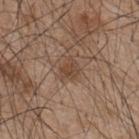biopsy_status: not biopsied; imaged during a skin examination
automated_metrics:
  area_mm2_approx: 5.0
  eccentricity: 0.45
  shape_asymmetry: 0.45
  vs_skin_contrast_norm: 6.5
lighting: white-light
lesion_size:
  long_diameter_mm_approx: 3.0
patient:
  sex: male
  age_approx: 45
site: back
image:
  source: total-body photography crop
  field_of_view_mm: 15